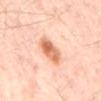biopsy status: total-body-photography surveillance lesion; no biopsy
automated metrics: a lesion color around L≈69 a*≈25 b*≈36 in CIELAB, about 14 CIELAB-L* units darker than the surrounding skin, and a normalized lesion–skin contrast near 8.5
subject: aged approximately 55
image source: total-body-photography crop, ~15 mm field of view
body site: the back